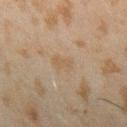Impression:
Part of a total-body skin-imaging series; this lesion was reviewed on a skin check and was not flagged for biopsy.
Clinical summary:
About 3 mm across. A male subject approximately 45 years of age. A 15 mm close-up tile from a total-body photography series done for melanoma screening. On the right forearm. This is a cross-polarized tile.